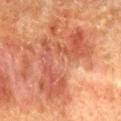No biopsy was performed on this lesion — it was imaged during a full skin examination and was not determined to be concerning.
From the mid back.
About 9.5 mm across.
A lesion tile, about 15 mm wide, cut from a 3D total-body photograph.
The lesion-visualizer software estimated an outline eccentricity of about 0.85 (0 = round, 1 = elongated).
The patient is a male aged around 50.